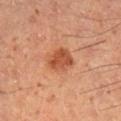{"biopsy_status": "not biopsied; imaged during a skin examination", "patient": {"sex": "male", "age_approx": 50}, "site": "right lower leg", "lighting": "cross-polarized", "automated_metrics": {"eccentricity": 0.7, "shape_asymmetry": 0.2, "cielab_L": 49, "cielab_a": 28, "cielab_b": 35, "vs_skin_darker_L": 11.0, "vs_skin_contrast_norm": 8.0, "border_irregularity_0_10": 2.0, "color_variation_0_10": 3.0, "peripheral_color_asymmetry": 1.0, "nevus_likeness_0_100": 90, "lesion_detection_confidence_0_100": 100}, "image": {"source": "total-body photography crop", "field_of_view_mm": 15}, "lesion_size": {"long_diameter_mm_approx": 3.5}}